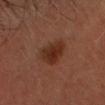Q: Was this lesion biopsied?
A: imaged on a skin check; not biopsied
Q: How was the tile lit?
A: cross-polarized illumination
Q: What did automated image analysis measure?
A: a shape eccentricity near 0.45 and a shape-asymmetry score of about 0.2 (0 = symmetric); an average lesion color of about L≈28 a*≈21 b*≈27 (CIELAB), a lesion–skin lightness drop of about 8, and a lesion-to-skin contrast of about 8.5 (normalized; higher = more distinct); border irregularity of about 2 on a 0–10 scale and a within-lesion color-variation index near 3.5/10; a lesion-detection confidence of about 100/100
Q: What are the patient's age and sex?
A: male, in their mid-40s
Q: What is the lesion's diameter?
A: about 3.5 mm
Q: What is the imaging modality?
A: ~15 mm crop, total-body skin-cancer survey
Q: Lesion location?
A: the head or neck